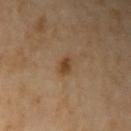<tbp_lesion>
  <biopsy_status>not biopsied; imaged during a skin examination</biopsy_status>
  <automated_metrics>
    <border_irregularity_0_10>2.0</border_irregularity_0_10>
    <color_variation_0_10>1.5</color_variation_0_10>
    <lesion_detection_confidence_0_100>100</lesion_detection_confidence_0_100>
  </automated_metrics>
  <image>
    <source>total-body photography crop</source>
    <field_of_view_mm>15</field_of_view_mm>
  </image>
  <lesion_size>
    <long_diameter_mm_approx>2.0</long_diameter_mm_approx>
  </lesion_size>
  <lighting>cross-polarized</lighting>
  <site>left upper arm</site>
  <patient>
    <sex>female</sex>
    <age_approx>65</age_approx>
  </patient>
</tbp_lesion>